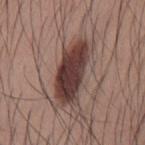| field | value |
|---|---|
| workup | catalogued during a skin exam; not biopsied |
| subject | male, aged approximately 35 |
| body site | the back |
| acquisition | ~15 mm tile from a whole-body skin photo |
| image-analysis metrics | a mean CIELAB color near L≈38 a*≈19 b*≈20, a lesion–skin lightness drop of about 16, and a normalized lesion–skin contrast near 13; border irregularity of about 3 on a 0–10 scale and peripheral color asymmetry of about 1.5; an automated nevus-likeness rating near 95 out of 100 and a lesion-detection confidence of about 100/100 |
| lighting | white-light |